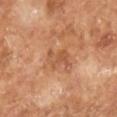Case summary:
• follow-up: no biopsy performed (imaged during a skin exam)
• image source: ~15 mm crop, total-body skin-cancer survey
• patient: male, aged 63 to 67
• lesion size: ~4 mm (longest diameter)
• automated metrics: a lesion area of about 6 mm², an outline eccentricity of about 0.8 (0 = round, 1 = elongated), and a symmetry-axis asymmetry near 0.45; a lesion color around L≈52 a*≈24 b*≈35 in CIELAB, roughly 7 lightness units darker than nearby skin, and a normalized border contrast of about 5.5
• lighting: cross-polarized